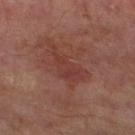Assessment:
Imaged during a routine full-body skin examination; the lesion was not biopsied and no histopathology is available.
Image and clinical context:
A male patient aged 63–67. The lesion is located on the left thigh. Captured under cross-polarized illumination. Measured at roughly 5.5 mm in maximum diameter. A close-up tile cropped from a whole-body skin photograph, about 15 mm across.